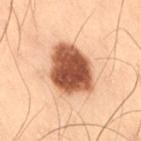Captured under cross-polarized illumination. A roughly 15 mm field-of-view crop from a total-body skin photograph. The lesion-visualizer software estimated a lesion color around L≈44 a*≈21 b*≈29 in CIELAB, about 20 CIELAB-L* units darker than the surrounding skin, and a normalized border contrast of about 14. The analysis additionally found a border-irregularity index near 2/10, a color-variation rating of about 5/10, and radial color variation of about 1.5. The analysis additionally found an automated nevus-likeness rating near 100 out of 100 and a lesion-detection confidence of about 100/100. A male subject, aged around 55. From the right thigh. The lesion's longest dimension is about 5.5 mm.